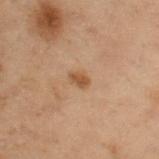Q: Is there a histopathology result?
A: no biopsy performed (imaged during a skin exam)
Q: What lighting was used for the tile?
A: cross-polarized illumination
Q: Where on the body is the lesion?
A: the arm
Q: What are the patient's age and sex?
A: female, in their mid- to late 50s
Q: How large is the lesion?
A: ≈2.5 mm
Q: What kind of image is this?
A: 15 mm crop, total-body photography